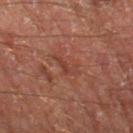Part of a total-body skin-imaging series; this lesion was reviewed on a skin check and was not flagged for biopsy. The lesion is on the left thigh. A lesion tile, about 15 mm wide, cut from a 3D total-body photograph. A male subject, aged approximately 70. The recorded lesion diameter is about 3.5 mm. Imaged with cross-polarized lighting. Automated tile analysis of the lesion measured border irregularity of about 6 on a 0–10 scale, a color-variation rating of about 0/10, and peripheral color asymmetry of about 0.